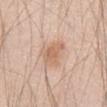{
  "biopsy_status": "not biopsied; imaged during a skin examination",
  "patient": {
    "sex": "male",
    "age_approx": 45
  },
  "image": {
    "source": "total-body photography crop",
    "field_of_view_mm": 15
  },
  "lighting": "white-light",
  "automated_metrics": {
    "cielab_L": 63,
    "cielab_a": 20,
    "cielab_b": 32,
    "vs_skin_darker_L": 9.0,
    "vs_skin_contrast_norm": 6.5,
    "color_variation_0_10": 2.0,
    "peripheral_color_asymmetry": 1.0,
    "nevus_likeness_0_100": 75,
    "lesion_detection_confidence_0_100": 100
  },
  "site": "abdomen"
}